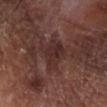workup=catalogued during a skin exam; not biopsied
diameter=~4 mm (longest diameter)
acquisition=~15 mm crop, total-body skin-cancer survey
automated metrics=a normalized border contrast of about 6.5; border irregularity of about 4.5 on a 0–10 scale; a nevus-likeness score of about 0/100 and lesion-presence confidence of about 95/100
subject=male, aged 68–72
anatomic site=the right lower leg
lighting=cross-polarized illumination